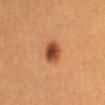<tbp_lesion>
<biopsy_status>not biopsied; imaged during a skin examination</biopsy_status>
<site>chest</site>
<lesion_size>
  <long_diameter_mm_approx>3.0</long_diameter_mm_approx>
</lesion_size>
<image>
  <source>total-body photography crop</source>
  <field_of_view_mm>15</field_of_view_mm>
</image>
<patient>
  <sex>female</sex>
  <age_approx>30</age_approx>
</patient>
</tbp_lesion>